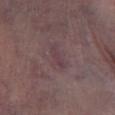• follow-up: no biopsy performed (imaged during a skin exam)
• image: 15 mm crop, total-body photography
• lesion size: about 3 mm
• image-analysis metrics: a lesion area of about 3.5 mm² and a symmetry-axis asymmetry near 0.3; border irregularity of about 4 on a 0–10 scale, a within-lesion color-variation index near 0/10, and radial color variation of about 0
• illumination: white-light illumination
• site: the left lower leg
• patient: male, aged 68 to 72